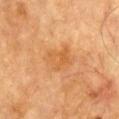Clinical impression: Captured during whole-body skin photography for melanoma surveillance; the lesion was not biopsied. Context: Automated image analysis of the tile measured a footprint of about 9.5 mm², a shape eccentricity near 0.55, and a shape-asymmetry score of about 0.3 (0 = symmetric). The analysis additionally found a border-irregularity rating of about 3/10 and a peripheral color-asymmetry measure near 1. A male subject, aged around 85 or older. The lesion is located on the chest. A lesion tile, about 15 mm wide, cut from a 3D total-body photograph.